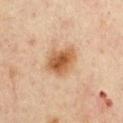Part of a total-body skin-imaging series; this lesion was reviewed on a skin check and was not flagged for biopsy. The lesion is located on the front of the torso. A female subject, aged 43–47. Cropped from a whole-body photographic skin survey; the tile spans about 15 mm. This is a cross-polarized tile.